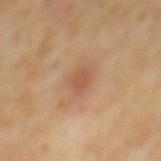| key | value |
|---|---|
| follow-up | catalogued during a skin exam; not biopsied |
| body site | the mid back |
| subject | male, about 55 years old |
| acquisition | ~15 mm tile from a whole-body skin photo |